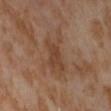Q: How was this image acquired?
A: ~15 mm crop, total-body skin-cancer survey
Q: Who is the patient?
A: female, in their mid- to late 50s
Q: Automated lesion metrics?
A: border irregularity of about 6.5 on a 0–10 scale and a color-variation rating of about 2.5/10; a nevus-likeness score of about 0/100
Q: How was the tile lit?
A: cross-polarized illumination
Q: Lesion location?
A: the leg